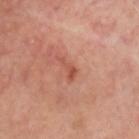workup — total-body-photography surveillance lesion; no biopsy
lesion diameter — ≈3 mm
illumination — cross-polarized illumination
anatomic site — the head or neck
subject — male, approximately 65 years of age
imaging modality — 15 mm crop, total-body photography